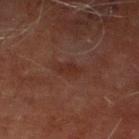Q: Was a biopsy performed?
A: total-body-photography surveillance lesion; no biopsy
Q: What is the anatomic site?
A: the right lower leg
Q: What is the lesion's diameter?
A: ~4 mm (longest diameter)
Q: What kind of image is this?
A: total-body-photography crop, ~15 mm field of view
Q: Patient demographics?
A: male, aged approximately 70